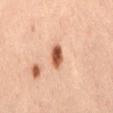{
  "biopsy_status": "not biopsied; imaged during a skin examination",
  "lesion_size": {
    "long_diameter_mm_approx": 3.0
  },
  "patient": {
    "sex": "female",
    "age_approx": 45
  },
  "lighting": "cross-polarized",
  "automated_metrics": {
    "border_irregularity_0_10": 1.5,
    "color_variation_0_10": 5.5
  },
  "site": "right thigh",
  "image": {
    "source": "total-body photography crop",
    "field_of_view_mm": 15
  }
}